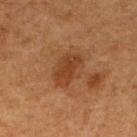Findings:
• notes — catalogued during a skin exam; not biopsied
• site — the upper back
• lesion diameter — ≈4.5 mm
• patient — male, approximately 65 years of age
• automated metrics — a shape eccentricity near 0.75; a color-variation rating of about 2.5/10 and radial color variation of about 1
• illumination — cross-polarized
• image — total-body-photography crop, ~15 mm field of view